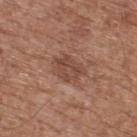Part of a total-body skin-imaging series; this lesion was reviewed on a skin check and was not flagged for biopsy. The tile uses white-light illumination. A region of skin cropped from a whole-body photographic capture, roughly 15 mm wide. The patient is a male aged approximately 75. From the upper back. The total-body-photography lesion software estimated a lesion area of about 7 mm², an outline eccentricity of about 0.65 (0 = round, 1 = elongated), and a shape-asymmetry score of about 0.3 (0 = symmetric). The software also gave border irregularity of about 3 on a 0–10 scale and a peripheral color-asymmetry measure near 1.5. The software also gave a classifier nevus-likeness of about 0/100 and a lesion-detection confidence of about 100/100. The lesion's longest dimension is about 3.5 mm.